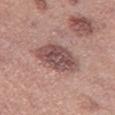Imaged during a routine full-body skin examination; the lesion was not biopsied and no histopathology is available. From the left thigh. A female subject, aged around 40. A close-up tile cropped from a whole-body skin photograph, about 15 mm across.